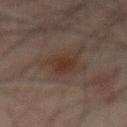The recorded lesion diameter is about 4 mm. The lesion is located on the back. A male subject aged approximately 70. Cropped from a whole-body photographic skin survey; the tile spans about 15 mm. The tile uses cross-polarized illumination.